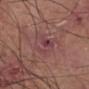biopsy status: total-body-photography surveillance lesion; no biopsy
patient: male, approximately 45 years of age
lighting: white-light illumination
TBP lesion metrics: a lesion–skin lightness drop of about 6 and a normalized lesion–skin contrast near 6.5; an automated nevus-likeness rating near 0 out of 100
size: ~5 mm (longest diameter)
location: the leg
image source: 15 mm crop, total-body photography